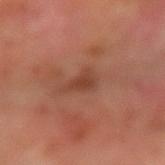The subject is a male in their 50s. A roughly 15 mm field-of-view crop from a total-body skin photograph. The lesion is on the left arm.